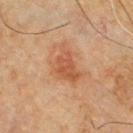This lesion was catalogued during total-body skin photography and was not selected for biopsy.
A lesion tile, about 15 mm wide, cut from a 3D total-body photograph.
Located on the front of the torso.
The total-body-photography lesion software estimated a footprint of about 9.5 mm² and an outline eccentricity of about 0.75 (0 = round, 1 = elongated). The analysis additionally found a border-irregularity index near 5/10, internal color variation of about 3 on a 0–10 scale, and radial color variation of about 1. It also reported a classifier nevus-likeness of about 50/100 and a detector confidence of about 100 out of 100 that the crop contains a lesion.
This is a cross-polarized tile.
A male subject approximately 65 years of age.
Measured at roughly 4.5 mm in maximum diameter.